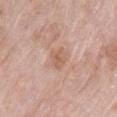The lesion was tiled from a total-body skin photograph and was not biopsied.
Cropped from a whole-body photographic skin survey; the tile spans about 15 mm.
Approximately 3 mm at its widest.
The patient is a male aged approximately 75.
Automated tile analysis of the lesion measured an average lesion color of about L≈60 a*≈20 b*≈29 (CIELAB), roughly 7 lightness units darker than nearby skin, and a normalized lesion–skin contrast near 5.5. The software also gave a lesion-detection confidence of about 100/100.
This is a white-light tile.
On the front of the torso.